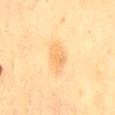The lesion was photographed on a routine skin check and not biopsied; there is no pathology result. Automated tile analysis of the lesion measured a mean CIELAB color near L≈65 a*≈17 b*≈40, about 7 CIELAB-L* units darker than the surrounding skin, and a normalized border contrast of about 5. The analysis additionally found border irregularity of about 2.5 on a 0–10 scale, a color-variation rating of about 2.5/10, and radial color variation of about 1. It also reported a classifier nevus-likeness of about 10/100. Located on the back. The tile uses cross-polarized illumination. A lesion tile, about 15 mm wide, cut from a 3D total-body photograph. The recorded lesion diameter is about 4 mm. A female patient, aged around 55.